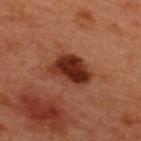Clinical impression:
The lesion was tiled from a total-body skin photograph and was not biopsied.
Clinical summary:
Cropped from a total-body skin-imaging series; the visible field is about 15 mm. Located on the upper back. Imaged with cross-polarized lighting. The patient is a male aged 48–52. The recorded lesion diameter is about 5 mm.